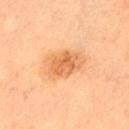The lesion is located on the lower back.
A female patient, roughly 45 years of age.
Captured under cross-polarized illumination.
Measured at roughly 5 mm in maximum diameter.
An algorithmic analysis of the crop reported internal color variation of about 5 on a 0–10 scale and a peripheral color-asymmetry measure near 1.5.
Cropped from a whole-body photographic skin survey; the tile spans about 15 mm.